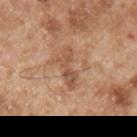Imaged during a routine full-body skin examination; the lesion was not biopsied and no histopathology is available. The tile uses white-light illumination. A male patient in their mid-50s. Longest diameter approximately 4.5 mm. From the left upper arm. Cropped from a whole-body photographic skin survey; the tile spans about 15 mm.